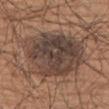biopsy_status: not biopsied; imaged during a skin examination
patient:
  sex: male
  age_approx: 75
automated_metrics:
  border_irregularity_0_10: 2.5
  color_variation_0_10: 6.5
  peripheral_color_asymmetry: 2.0
image:
  source: total-body photography crop
  field_of_view_mm: 15
site: upper back
lighting: white-light